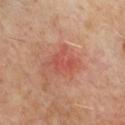The lesion was tiled from a total-body skin photograph and was not biopsied. The lesion is on the chest. A male patient aged 63–67. A 15 mm close-up tile from a total-body photography series done for melanoma screening. The lesion-visualizer software estimated a lesion color around L≈50 a*≈29 b*≈29 in CIELAB, a lesion–skin lightness drop of about 7, and a lesion-to-skin contrast of about 5.5 (normalized; higher = more distinct). And it measured a border-irregularity index near 5.5/10 and a peripheral color-asymmetry measure near 0.5. It also reported a nevus-likeness score of about 5/100 and a lesion-detection confidence of about 100/100. The tile uses cross-polarized illumination.